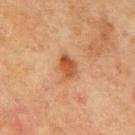{
  "lesion_size": {
    "long_diameter_mm_approx": 3.0
  },
  "site": "back",
  "image": {
    "source": "total-body photography crop",
    "field_of_view_mm": 15
  },
  "automated_metrics": {
    "color_variation_0_10": 3.5,
    "nevus_likeness_0_100": 70,
    "lesion_detection_confidence_0_100": 100
  },
  "patient": {
    "sex": "male",
    "age_approx": 70
  }
}